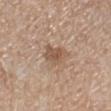notes — imaged on a skin check; not biopsied
anatomic site — the leg
imaging modality — 15 mm crop, total-body photography
automated lesion analysis — an area of roughly 6 mm², an outline eccentricity of about 0.65 (0 = round, 1 = elongated), and a shape-asymmetry score of about 0.35 (0 = symmetric); a nevus-likeness score of about 40/100 and a lesion-detection confidence of about 100/100
patient — female, aged around 60